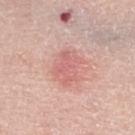Q: Was this lesion biopsied?
A: imaged on a skin check; not biopsied
Q: Who is the patient?
A: male, aged approximately 70
Q: How was this image acquired?
A: ~15 mm crop, total-body skin-cancer survey
Q: Lesion location?
A: the right lower leg